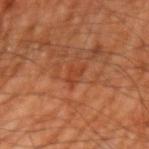Captured during whole-body skin photography for melanoma surveillance; the lesion was not biopsied. Measured at roughly 3 mm in maximum diameter. Cropped from a whole-body photographic skin survey; the tile spans about 15 mm. This is a cross-polarized tile. The subject is a male aged 58 to 62. On the left upper arm. An algorithmic analysis of the crop reported an average lesion color of about L≈41 a*≈28 b*≈35 (CIELAB), roughly 6 lightness units darker than nearby skin, and a normalized border contrast of about 5.